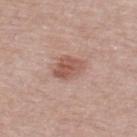The lesion was photographed on a routine skin check and not biopsied; there is no pathology result. Located on the upper back. Captured under white-light illumination. The lesion-visualizer software estimated a lesion area of about 7.5 mm², an outline eccentricity of about 0.65 (0 = round, 1 = elongated), and a symmetry-axis asymmetry near 0.25. And it measured a mean CIELAB color near L≈54 a*≈23 b*≈26, about 11 CIELAB-L* units darker than the surrounding skin, and a normalized lesion–skin contrast near 7.5. The analysis additionally found internal color variation of about 3.5 on a 0–10 scale and peripheral color asymmetry of about 1.5. A 15 mm crop from a total-body photograph taken for skin-cancer surveillance. The lesion's longest dimension is about 3.5 mm. A male patient, in their mid-50s.